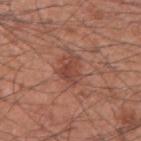Impression:
The lesion was tiled from a total-body skin photograph and was not biopsied.
Image and clinical context:
A close-up tile cropped from a whole-body skin photograph, about 15 mm across. The lesion is located on the right upper arm. A male subject, roughly 60 years of age.